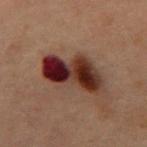workup: catalogued during a skin exam; not biopsied
illumination: cross-polarized illumination
size: about 6 mm
location: the mid back
automated metrics: an area of roughly 18 mm², a shape eccentricity near 0.8, and a symmetry-axis asymmetry near 0.3; a detector confidence of about 100 out of 100 that the crop contains a lesion
patient: male, in their 60s
image: ~15 mm crop, total-body skin-cancer survey